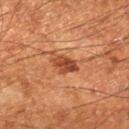<record>
<biopsy_status>not biopsied; imaged during a skin examination</biopsy_status>
<site>right lower leg</site>
<automated_metrics>
  <cielab_L>44</cielab_L>
  <cielab_a>28</cielab_a>
  <cielab_b>35</cielab_b>
  <vs_skin_darker_L>12.0</vs_skin_darker_L>
  <vs_skin_contrast_norm>9.0</vs_skin_contrast_norm>
  <border_irregularity_0_10>2.5</border_irregularity_0_10>
  <color_variation_0_10>4.5</color_variation_0_10>
</automated_metrics>
<patient>
  <sex>male</sex>
  <age_approx>45</age_approx>
</patient>
<image>
  <source>total-body photography crop</source>
  <field_of_view_mm>15</field_of_view_mm>
</image>
<lighting>cross-polarized</lighting>
<lesion_size>
  <long_diameter_mm_approx>3.0</long_diameter_mm_approx>
</lesion_size>
</record>